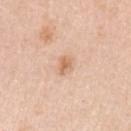workup = total-body-photography surveillance lesion; no biopsy | patient = female, aged approximately 45 | image source = 15 mm crop, total-body photography | body site = the left upper arm | illumination = white-light illumination | image-analysis metrics = a border-irregularity rating of about 2/10 and internal color variation of about 4 on a 0–10 scale.